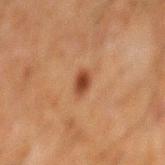biopsy_status: not biopsied; imaged during a skin examination
patient:
  sex: male
  age_approx: 60
image:
  source: total-body photography crop
  field_of_view_mm: 15
site: mid back